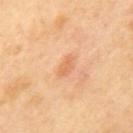Assessment: The lesion was photographed on a routine skin check and not biopsied; there is no pathology result. Context: A 15 mm close-up tile from a total-body photography series done for melanoma screening. Located on the mid back.